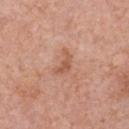Assessment: Recorded during total-body skin imaging; not selected for excision or biopsy. Background: The lesion is located on the chest. A male subject, approximately 70 years of age. A lesion tile, about 15 mm wide, cut from a 3D total-body photograph. Automated image analysis of the tile measured roughly 9 lightness units darker than nearby skin and a normalized border contrast of about 6. The analysis additionally found a border-irregularity rating of about 6/10, internal color variation of about 1.5 on a 0–10 scale, and peripheral color asymmetry of about 0.5. Captured under white-light illumination. About 3 mm across.